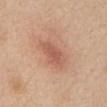{
  "biopsy_status": "not biopsied; imaged during a skin examination",
  "lighting": "white-light",
  "site": "abdomen",
  "lesion_size": {
    "long_diameter_mm_approx": 3.5
  },
  "automated_metrics": {
    "area_mm2_approx": 6.0,
    "eccentricity": 0.8,
    "shape_asymmetry": 0.25,
    "vs_skin_darker_L": 8.0,
    "vs_skin_contrast_norm": 5.5,
    "border_irregularity_0_10": 2.5,
    "color_variation_0_10": 2.0,
    "peripheral_color_asymmetry": 0.5,
    "nevus_likeness_0_100": 60,
    "lesion_detection_confidence_0_100": 100
  },
  "image": {
    "source": "total-body photography crop",
    "field_of_view_mm": 15
  },
  "patient": {
    "sex": "female",
    "age_approx": 70
  }
}